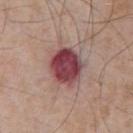  biopsy_status: not biopsied; imaged during a skin examination
  lighting: white-light
  patient:
    sex: male
    age_approx: 50
  site: chest
  image:
    source: total-body photography crop
    field_of_view_mm: 15
  lesion_size:
    long_diameter_mm_approx: 4.5